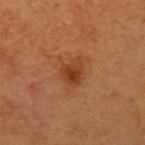Assessment:
Captured during whole-body skin photography for melanoma surveillance; the lesion was not biopsied.
Background:
A roughly 15 mm field-of-view crop from a total-body skin photograph. The subject is a male aged approximately 75. The lesion is on the arm.